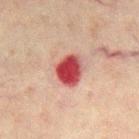biopsy status = total-body-photography surveillance lesion; no biopsy | lesion diameter = ~3.5 mm (longest diameter) | automated metrics = a lesion area of about 9.5 mm² and an eccentricity of roughly 0.5; a lesion color around L≈38 a*≈29 b*≈21 in CIELAB, about 15 CIELAB-L* units darker than the surrounding skin, and a normalized lesion–skin contrast near 12; a border-irregularity rating of about 2/10, internal color variation of about 6.5 on a 0–10 scale, and a peripheral color-asymmetry measure near 2; a lesion-detection confidence of about 100/100 | subject = male, approximately 60 years of age | imaging modality = ~15 mm crop, total-body skin-cancer survey | illumination = cross-polarized illumination | body site = the chest.